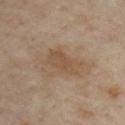Impression:
Imaged during a routine full-body skin examination; the lesion was not biopsied and no histopathology is available.
Acquisition and patient details:
The lesion-visualizer software estimated a mean CIELAB color near L≈50 a*≈15 b*≈31, roughly 7 lightness units darker than nearby skin, and a lesion-to-skin contrast of about 6 (normalized; higher = more distinct). The analysis additionally found a border-irregularity index near 3/10, internal color variation of about 3 on a 0–10 scale, and peripheral color asymmetry of about 1. And it measured a classifier nevus-likeness of about 0/100. On the chest. The tile uses cross-polarized illumination. Cropped from a whole-body photographic skin survey; the tile spans about 15 mm. A male subject about 55 years old.